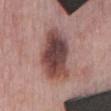Clinical summary: The subject is a male roughly 75 years of age. From the mid back. Cropped from a whole-body photographic skin survey; the tile spans about 15 mm. Diagnosis: Histopathology of the biopsied lesion showed a dysplastic (Clark) nevus — a benign skin lesion.